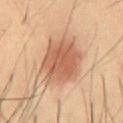Notes:
– notes · total-body-photography surveillance lesion; no biopsy
– body site · the abdomen
– imaging modality · 15 mm crop, total-body photography
– subject · male, roughly 55 years of age